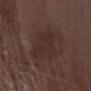Cropped from a total-body skin-imaging series; the visible field is about 15 mm. A male patient, aged 68–72. Measured at roughly 6 mm in maximum diameter. Located on the right forearm.